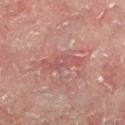patient:
  sex: male
  age_approx: 60
lesion_size:
  long_diameter_mm_approx: 5.0
site: left lower leg
lighting: cross-polarized
image:
  source: total-body photography crop
  field_of_view_mm: 15